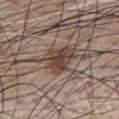Clinical summary:
On the chest. This is a white-light tile. The lesion-visualizer software estimated an area of roughly 10 mm², a shape eccentricity near 0.55, and a shape-asymmetry score of about 0.3 (0 = symmetric). A lesion tile, about 15 mm wide, cut from a 3D total-body photograph. The subject is a male aged 58 to 62.